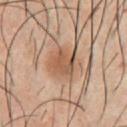The lesion was photographed on a routine skin check and not biopsied; there is no pathology result.
On the chest.
About 3.5 mm across.
This is a cross-polarized tile.
A 15 mm close-up tile from a total-body photography series done for melanoma screening.
The patient is a male aged approximately 40.